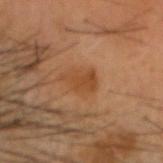Located on the head or neck. A male patient, aged approximately 55. The tile uses cross-polarized illumination. A 15 mm close-up tile from a total-body photography series done for melanoma screening.